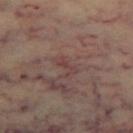The lesion was photographed on a routine skin check and not biopsied; there is no pathology result. A subject aged 58–62. This is a cross-polarized tile. The lesion is on the left thigh. Cropped from a whole-body photographic skin survey; the tile spans about 15 mm.